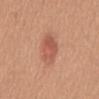Imaged during a routine full-body skin examination; the lesion was not biopsied and no histopathology is available. From the back. Measured at roughly 4 mm in maximum diameter. A female subject aged 53–57. The tile uses white-light illumination. A roughly 15 mm field-of-view crop from a total-body skin photograph. The total-body-photography lesion software estimated a footprint of about 9 mm², an eccentricity of roughly 0.7, and a symmetry-axis asymmetry near 0.15.